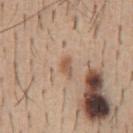Assessment: Part of a total-body skin-imaging series; this lesion was reviewed on a skin check and was not flagged for biopsy.